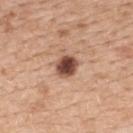Impression:
This lesion was catalogued during total-body skin photography and was not selected for biopsy.
Clinical summary:
A female patient aged 53 to 57. Measured at roughly 3 mm in maximum diameter. A 15 mm close-up extracted from a 3D total-body photography capture. On the upper back.